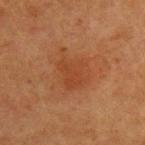| field | value |
|---|---|
| follow-up | no biopsy performed (imaged during a skin exam) |
| site | the upper back |
| tile lighting | cross-polarized |
| lesion size | ≈4.5 mm |
| patient | male, aged 63–67 |
| image | total-body-photography crop, ~15 mm field of view |